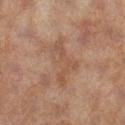A 15 mm close-up extracted from a 3D total-body photography capture. The lesion is located on the right lower leg. The patient is a female aged approximately 60. Longest diameter approximately 6 mm.